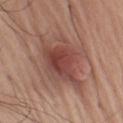  biopsy_status: not biopsied; imaged during a skin examination
  site: left upper arm
  patient:
    sex: male
    age_approx: 70
  image:
    source: total-body photography crop
    field_of_view_mm: 15